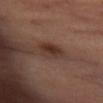Notes:
- biopsy status · total-body-photography surveillance lesion; no biopsy
- automated metrics · a mean CIELAB color near L≈34 a*≈19 b*≈24, about 10 CIELAB-L* units darker than the surrounding skin, and a lesion-to-skin contrast of about 8.5 (normalized; higher = more distinct); an automated nevus-likeness rating near 85 out of 100 and a detector confidence of about 100 out of 100 that the crop contains a lesion
- site · the abdomen
- imaging modality · 15 mm crop, total-body photography
- lighting · cross-polarized illumination
- patient · female, about 55 years old